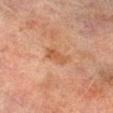| field | value |
|---|---|
| workup | total-body-photography surveillance lesion; no biopsy |
| body site | the leg |
| automated lesion analysis | an area of roughly 4 mm² and two-axis asymmetry of about 0.25; a normalized border contrast of about 6.5; border irregularity of about 2.5 on a 0–10 scale and internal color variation of about 1.5 on a 0–10 scale; a nevus-likeness score of about 5/100 and a detector confidence of about 100 out of 100 that the crop contains a lesion |
| tile lighting | cross-polarized illumination |
| image | ~15 mm crop, total-body skin-cancer survey |
| patient | male, aged around 75 |
| lesion size | about 2.5 mm |